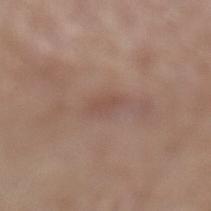{"biopsy_status": "not biopsied; imaged during a skin examination", "image": {"source": "total-body photography crop", "field_of_view_mm": 15}, "lesion_size": {"long_diameter_mm_approx": 3.0}, "patient": {"sex": "female", "age_approx": 65}, "automated_metrics": {"cielab_L": 50, "cielab_a": 18, "cielab_b": 24, "border_irregularity_0_10": 2.5, "color_variation_0_10": 1.0, "peripheral_color_asymmetry": 0.5}, "site": "leg", "lighting": "white-light"}